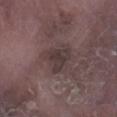| feature | finding |
|---|---|
| workup | catalogued during a skin exam; not biopsied |
| anatomic site | the right lower leg |
| subject | male, about 75 years old |
| lesion size | ≈3.5 mm |
| automated lesion analysis | a shape-asymmetry score of about 0.45 (0 = symmetric); a normalized border contrast of about 7; a border-irregularity index near 4/10, a color-variation rating of about 3.5/10, and radial color variation of about 1 |
| illumination | white-light illumination |
| imaging modality | ~15 mm crop, total-body skin-cancer survey |